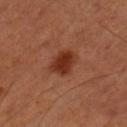Recorded during total-body skin imaging; not selected for excision or biopsy.
The lesion-visualizer software estimated a footprint of about 7.5 mm², a shape eccentricity near 0.7, and two-axis asymmetry of about 0.2. It also reported a lesion color around L≈34 a*≈27 b*≈32 in CIELAB, a lesion–skin lightness drop of about 11, and a normalized lesion–skin contrast near 9.5. And it measured a border-irregularity rating of about 2/10, a within-lesion color-variation index near 2.5/10, and a peripheral color-asymmetry measure near 1. The software also gave a nevus-likeness score of about 95/100.
About 3.5 mm across.
A region of skin cropped from a whole-body photographic capture, roughly 15 mm wide.
Captured under cross-polarized illumination.
The lesion is on the left upper arm.
The subject is a male aged 48–52.